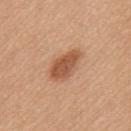Part of a total-body skin-imaging series; this lesion was reviewed on a skin check and was not flagged for biopsy. A roughly 15 mm field-of-view crop from a total-body skin photograph. The subject is a female aged approximately 45. From the mid back.